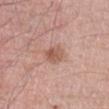No biopsy was performed on this lesion — it was imaged during a full skin examination and was not determined to be concerning.
A 15 mm close-up extracted from a 3D total-body photography capture.
The lesion-visualizer software estimated a mean CIELAB color near L≈56 a*≈22 b*≈28, roughly 10 lightness units darker than nearby skin, and a normalized border contrast of about 6.5. The analysis additionally found border irregularity of about 1.5 on a 0–10 scale, internal color variation of about 3.5 on a 0–10 scale, and radial color variation of about 1.5.
A male patient roughly 70 years of age.
The lesion is located on the front of the torso.